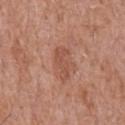No biopsy was performed on this lesion — it was imaged during a full skin examination and was not determined to be concerning.
A close-up tile cropped from a whole-body skin photograph, about 15 mm across.
Approximately 4 mm at its widest.
From the chest.
The subject is a male aged 63 to 67.